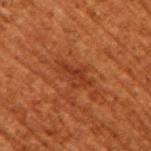Impression: No biopsy was performed on this lesion — it was imaged during a full skin examination and was not determined to be concerning. Background: The lesion's longest dimension is about 4 mm. The lesion is located on the left upper arm. A roughly 15 mm field-of-view crop from a total-body skin photograph. A female subject aged around 80. Captured under cross-polarized illumination.